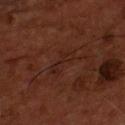Assessment:
Part of a total-body skin-imaging series; this lesion was reviewed on a skin check and was not flagged for biopsy.
Context:
A male subject, aged 53 to 57. A roughly 15 mm field-of-view crop from a total-body skin photograph. On the chest. The total-body-photography lesion software estimated about 4 CIELAB-L* units darker than the surrounding skin and a lesion-to-skin contrast of about 5 (normalized; higher = more distinct). And it measured internal color variation of about 0 on a 0–10 scale and a peripheral color-asymmetry measure near 0. It also reported a nevus-likeness score of about 0/100 and a lesion-detection confidence of about 55/100. Longest diameter approximately 4 mm.